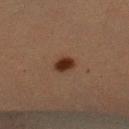Findings:
- follow-up · total-body-photography surveillance lesion; no biopsy
- patient · female, about 55 years old
- site · the left upper arm
- image source · ~15 mm tile from a whole-body skin photo